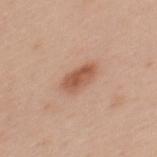Impression:
This lesion was catalogued during total-body skin photography and was not selected for biopsy.